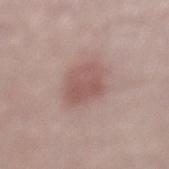Part of a total-body skin-imaging series; this lesion was reviewed on a skin check and was not flagged for biopsy.
The lesion is located on the back.
The tile uses white-light illumination.
The patient is a male approximately 70 years of age.
A 15 mm close-up tile from a total-body photography series done for melanoma screening.
Longest diameter approximately 4 mm.